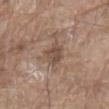Case summary:
- notes — total-body-photography surveillance lesion; no biopsy
- lesion size — about 3 mm
- illumination — white-light illumination
- acquisition — ~15 mm crop, total-body skin-cancer survey
- patient — male, roughly 80 years of age
- location — the abdomen